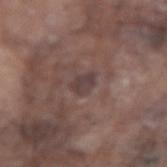notes: catalogued during a skin exam; not biopsied | image source: ~15 mm tile from a whole-body skin photo | automated lesion analysis: a mean CIELAB color near L≈39 a*≈16 b*≈17, roughly 7 lightness units darker than nearby skin, and a lesion-to-skin contrast of about 7 (normalized; higher = more distinct); border irregularity of about 2.5 on a 0–10 scale, a color-variation rating of about 2/10, and a peripheral color-asymmetry measure near 0.5 | tile lighting: white-light illumination | lesion diameter: about 2.5 mm | subject: male, aged around 75 | site: the arm.